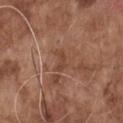The lesion was tiled from a total-body skin photograph and was not biopsied. The recorded lesion diameter is about 2.5 mm. Cropped from a total-body skin-imaging series; the visible field is about 15 mm. A male patient, roughly 75 years of age. Imaged with white-light lighting. On the front of the torso.